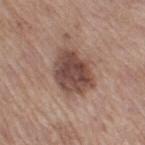Part of a total-body skin-imaging series; this lesion was reviewed on a skin check and was not flagged for biopsy.
The lesion is located on the right thigh.
A 15 mm close-up extracted from a 3D total-body photography capture.
The lesion's longest dimension is about 5.5 mm.
The tile uses white-light illumination.
The subject is a female in their mid-50s.